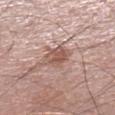No biopsy was performed on this lesion — it was imaged during a full skin examination and was not determined to be concerning. On the left lower leg. A male patient, aged around 55. The recorded lesion diameter is about 3.5 mm. Imaged with white-light lighting. Cropped from a whole-body photographic skin survey; the tile spans about 15 mm.